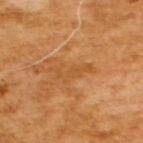notes = total-body-photography surveillance lesion; no biopsy | illumination = cross-polarized illumination | image = ~15 mm tile from a whole-body skin photo | size = ≈4.5 mm | subject = male, roughly 65 years of age.